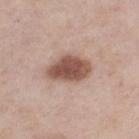Captured during whole-body skin photography for melanoma surveillance; the lesion was not biopsied.
A female subject, aged approximately 55.
Captured under white-light illumination.
Cropped from a whole-body photographic skin survey; the tile spans about 15 mm.
An algorithmic analysis of the crop reported border irregularity of about 2 on a 0–10 scale, internal color variation of about 3.5 on a 0–10 scale, and radial color variation of about 1.
Measured at roughly 5 mm in maximum diameter.
The lesion is on the left thigh.